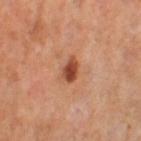Assessment:
The lesion was photographed on a routine skin check and not biopsied; there is no pathology result.
Clinical summary:
This is a cross-polarized tile. A 15 mm close-up tile from a total-body photography series done for melanoma screening. A female subject, aged 53–57. The lesion is located on the left thigh. Longest diameter approximately 3 mm.